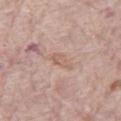| key | value |
|---|---|
| biopsy status | catalogued during a skin exam; not biopsied |
| patient | female, aged 68–72 |
| size | ~3 mm (longest diameter) |
| body site | the right thigh |
| imaging modality | ~15 mm crop, total-body skin-cancer survey |